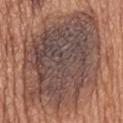– imaging modality: ~15 mm crop, total-body skin-cancer survey
– body site: the back
– image-analysis metrics: a mean CIELAB color near L≈46 a*≈17 b*≈22, roughly 11 lightness units darker than nearby skin, and a normalized lesion–skin contrast near 10; a detector confidence of about 95 out of 100 that the crop contains a lesion
– size: ≈14 mm
– subject: male, aged 63 to 67
– lighting: white-light illumination